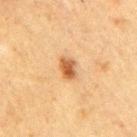Part of a total-body skin-imaging series; this lesion was reviewed on a skin check and was not flagged for biopsy. Captured under cross-polarized illumination. From the right upper arm. Longest diameter approximately 2.5 mm. Automated image analysis of the tile measured a lesion area of about 4.5 mm², an outline eccentricity of about 0.7 (0 = round, 1 = elongated), and two-axis asymmetry of about 0.2. The analysis additionally found a border-irregularity index near 2/10 and a within-lesion color-variation index near 4/10. It also reported a classifier nevus-likeness of about 95/100 and lesion-presence confidence of about 100/100. A 15 mm close-up extracted from a 3D total-body photography capture. The patient is a male in their 50s.